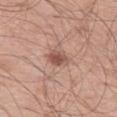The lesion was tiled from a total-body skin photograph and was not biopsied. Measured at roughly 3 mm in maximum diameter. The total-body-photography lesion software estimated an outline eccentricity of about 0.6 (0 = round, 1 = elongated). It also reported a lesion–skin lightness drop of about 11 and a lesion-to-skin contrast of about 8 (normalized; higher = more distinct). It also reported a color-variation rating of about 3.5/10 and a peripheral color-asymmetry measure near 1. It also reported an automated nevus-likeness rating near 90 out of 100 and a detector confidence of about 100 out of 100 that the crop contains a lesion. Cropped from a whole-body photographic skin survey; the tile spans about 15 mm. The tile uses white-light illumination. On the left thigh. The patient is a male approximately 70 years of age.